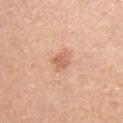Imaged during a routine full-body skin examination; the lesion was not biopsied and no histopathology is available. From the chest. A region of skin cropped from a whole-body photographic capture, roughly 15 mm wide. A male patient aged 48–52.